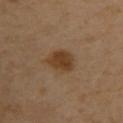Findings:
• workup · no biopsy performed (imaged during a skin exam)
• size · about 4 mm
• image · ~15 mm crop, total-body skin-cancer survey
• anatomic site · the upper back
• subject · female, in their 50s